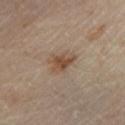Imaged during a routine full-body skin examination; the lesion was not biopsied and no histopathology is available.
The subject is a female roughly 45 years of age.
Automated tile analysis of the lesion measured border irregularity of about 3.5 on a 0–10 scale and a peripheral color-asymmetry measure near 1. The analysis additionally found an automated nevus-likeness rating near 60 out of 100 and lesion-presence confidence of about 100/100.
This is a cross-polarized tile.
Longest diameter approximately 3.5 mm.
The lesion is on the left lower leg.
A region of skin cropped from a whole-body photographic capture, roughly 15 mm wide.